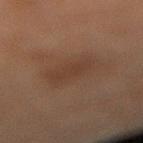- follow-up — catalogued during a skin exam; not biopsied
- lighting — cross-polarized
- acquisition — ~15 mm crop, total-body skin-cancer survey
- lesion diameter — about 4.5 mm
- location — the right lower leg
- subject — male, aged 68 to 72